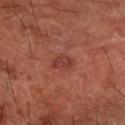biopsy_status: not biopsied; imaged during a skin examination
site: right forearm
image:
  source: total-body photography crop
  field_of_view_mm: 15
lighting: cross-polarized
lesion_size:
  long_diameter_mm_approx: 2.5
patient:
  sex: male
  age_approx: 60